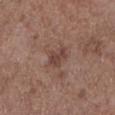Impression:
Imaged during a routine full-body skin examination; the lesion was not biopsied and no histopathology is available.
Acquisition and patient details:
From the left lower leg. A male subject roughly 60 years of age. The recorded lesion diameter is about 3 mm. This is a white-light tile. A 15 mm close-up tile from a total-body photography series done for melanoma screening. An algorithmic analysis of the crop reported a nevus-likeness score of about 0/100 and a lesion-detection confidence of about 100/100.